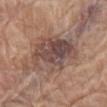follow-up: imaged on a skin check; not biopsied
image-analysis metrics: a lesion area of about 26 mm², a shape eccentricity near 0.8, and two-axis asymmetry of about 0.3; a border-irregularity index near 4.5/10, a color-variation rating of about 6.5/10, and radial color variation of about 2
patient: female, aged around 75
tile lighting: white-light illumination
diameter: ≈7.5 mm
location: the left thigh
image source: 15 mm crop, total-body photography